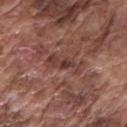<lesion>
  <biopsy_status>not biopsied; imaged during a skin examination</biopsy_status>
  <image>
    <source>total-body photography crop</source>
    <field_of_view_mm>15</field_of_view_mm>
  </image>
  <patient>
    <sex>male</sex>
    <age_approx>75</age_approx>
  </patient>
  <site>left upper arm</site>
  <lighting>white-light</lighting>
  <lesion_size>
    <long_diameter_mm_approx>4.5</long_diameter_mm_approx>
  </lesion_size>
</lesion>